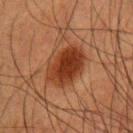biopsy status=total-body-photography surveillance lesion; no biopsy
illumination=cross-polarized
patient=male, aged approximately 50
diameter=about 5.5 mm
image=~15 mm tile from a whole-body skin photo
image-analysis metrics=a nevus-likeness score of about 100/100 and a detector confidence of about 100 out of 100 that the crop contains a lesion
anatomic site=the left upper arm